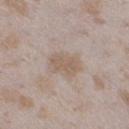notes: total-body-photography surveillance lesion; no biopsy | anatomic site: the left lower leg | image source: ~15 mm crop, total-body skin-cancer survey | lesion size: ≈4 mm | patient: female, aged 23–27.